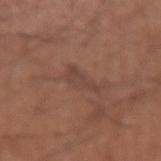Assessment: The lesion was photographed on a routine skin check and not biopsied; there is no pathology result. Image and clinical context: An algorithmic analysis of the crop reported border irregularity of about 6.5 on a 0–10 scale, internal color variation of about 1 on a 0–10 scale, and peripheral color asymmetry of about 0. A close-up tile cropped from a whole-body skin photograph, about 15 mm across. A male patient, approximately 65 years of age. The lesion is located on the right forearm.